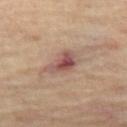Captured during whole-body skin photography for melanoma surveillance; the lesion was not biopsied.
Cropped from a total-body skin-imaging series; the visible field is about 15 mm.
This is a cross-polarized tile.
The lesion is located on the left thigh.
A female patient, about 65 years old.
An algorithmic analysis of the crop reported a footprint of about 7.5 mm², an eccentricity of roughly 0.85, and two-axis asymmetry of about 0.35. It also reported border irregularity of about 3.5 on a 0–10 scale, a within-lesion color-variation index near 7.5/10, and radial color variation of about 2.
About 4 mm across.